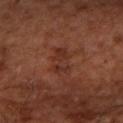Q: What lighting was used for the tile?
A: cross-polarized illumination
Q: What is the anatomic site?
A: the right upper arm
Q: Patient demographics?
A: aged approximately 65
Q: What kind of image is this?
A: 15 mm crop, total-body photography
Q: Lesion size?
A: ≈3.5 mm
Q: Automated lesion metrics?
A: a mean CIELAB color near L≈31 a*≈23 b*≈26 and about 6 CIELAB-L* units darker than the surrounding skin; lesion-presence confidence of about 100/100